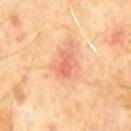biopsy status — catalogued during a skin exam; not biopsied
patient — male, roughly 60 years of age
illumination — cross-polarized
imaging modality — total-body-photography crop, ~15 mm field of view
body site — the front of the torso